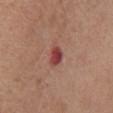{"automated_metrics": {"area_mm2_approx": 4.0, "eccentricity": 0.75, "cielab_L": 43, "cielab_a": 30, "cielab_b": 24, "vs_skin_darker_L": 13.0, "vs_skin_contrast_norm": 10.0, "border_irregularity_0_10": 2.5, "color_variation_0_10": 3.5, "nevus_likeness_0_100": 0, "lesion_detection_confidence_0_100": 100}, "patient": {"sex": "female", "age_approx": 75}, "image": {"source": "total-body photography crop", "field_of_view_mm": 15}, "lesion_size": {"long_diameter_mm_approx": 3.0}, "lighting": "white-light", "site": "chest"}